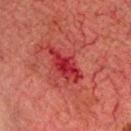This lesion was catalogued during total-body skin photography and was not selected for biopsy.
The lesion is located on the head or neck.
This image is a 15 mm lesion crop taken from a total-body photograph.
The recorded lesion diameter is about 5 mm.
A male subject roughly 60 years of age.
Automated tile analysis of the lesion measured a shape eccentricity near 0.85 and two-axis asymmetry of about 0.3. And it measured a border-irregularity index near 4/10, internal color variation of about 3.5 on a 0–10 scale, and a peripheral color-asymmetry measure near 1.